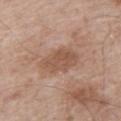This lesion was catalogued during total-body skin photography and was not selected for biopsy.